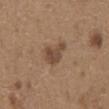Q: Where on the body is the lesion?
A: the front of the torso
Q: What are the patient's age and sex?
A: male, in their mid-60s
Q: How was this image acquired?
A: 15 mm crop, total-body photography